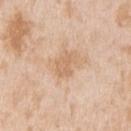No biopsy was performed on this lesion — it was imaged during a full skin examination and was not determined to be concerning.